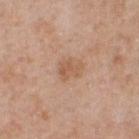| field | value |
|---|---|
| location | the chest |
| image | 15 mm crop, total-body photography |
| image-analysis metrics | a mean CIELAB color near L≈57 a*≈20 b*≈33, roughly 8 lightness units darker than nearby skin, and a lesion-to-skin contrast of about 6 (normalized; higher = more distinct); a border-irregularity index near 3.5/10, a within-lesion color-variation index near 2/10, and peripheral color asymmetry of about 1; a classifier nevus-likeness of about 0/100 and a detector confidence of about 100 out of 100 that the crop contains a lesion |
| lighting | white-light illumination |
| subject | male, roughly 50 years of age |